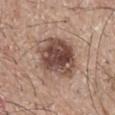Recorded during total-body skin imaging; not selected for excision or biopsy. The lesion is located on the back. Imaged with white-light lighting. Automated tile analysis of the lesion measured a within-lesion color-variation index near 7.5/10 and radial color variation of about 2.5. And it measured an automated nevus-likeness rating near 15 out of 100. A male patient, aged 63 to 67. The lesion's longest dimension is about 5.5 mm. A 15 mm close-up tile from a total-body photography series done for melanoma screening.